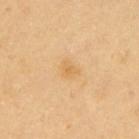biopsy status: no biopsy performed (imaged during a skin exam); illumination: cross-polarized; image: 15 mm crop, total-body photography; location: the back; patient: female, about 70 years old; diameter: ~2.5 mm (longest diameter).